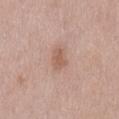Assessment: Part of a total-body skin-imaging series; this lesion was reviewed on a skin check and was not flagged for biopsy. Context: A female patient, approximately 60 years of age. A 15 mm crop from a total-body photograph taken for skin-cancer surveillance. The lesion is located on the lower back.